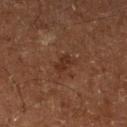Recorded during total-body skin imaging; not selected for excision or biopsy. Captured under cross-polarized illumination. From the left lower leg. Automated tile analysis of the lesion measured an area of roughly 3 mm², a shape eccentricity near 0.85, and two-axis asymmetry of about 0.4. A male subject, about 65 years old. Cropped from a total-body skin-imaging series; the visible field is about 15 mm.